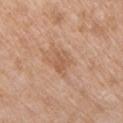Q: Was a biopsy performed?
A: imaged on a skin check; not biopsied
Q: Automated lesion metrics?
A: a lesion color around L≈57 a*≈21 b*≈34 in CIELAB and roughly 7 lightness units darker than nearby skin; a nevus-likeness score of about 0/100 and a lesion-detection confidence of about 100/100
Q: What kind of image is this?
A: ~15 mm tile from a whole-body skin photo
Q: Lesion size?
A: ~3 mm (longest diameter)
Q: What are the patient's age and sex?
A: male, about 65 years old
Q: What lighting was used for the tile?
A: white-light illumination
Q: What is the anatomic site?
A: the chest